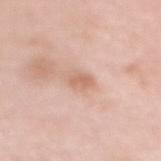Clinical impression:
This lesion was catalogued during total-body skin photography and was not selected for biopsy.
Context:
The tile uses white-light illumination. A female subject in their 70s. The lesion is located on the mid back. The recorded lesion diameter is about 2.5 mm. A roughly 15 mm field-of-view crop from a total-body skin photograph.